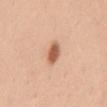biopsy_status: not biopsied; imaged during a skin examination
image:
  source: total-body photography crop
  field_of_view_mm: 15
site: mid back
patient:
  sex: female
  age_approx: 35